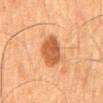workup — total-body-photography surveillance lesion; no biopsy | subject — male, in their mid-60s | location — the mid back | image — ~15 mm crop, total-body skin-cancer survey.